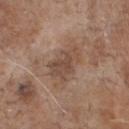Q: Was a biopsy performed?
A: catalogued during a skin exam; not biopsied
Q: What did automated image analysis measure?
A: an area of roughly 9.5 mm² and a shape-asymmetry score of about 0.35 (0 = symmetric); a mean CIELAB color near L≈47 a*≈17 b*≈26, about 8 CIELAB-L* units darker than the surrounding skin, and a normalized border contrast of about 6.5; a nevus-likeness score of about 0/100 and a lesion-detection confidence of about 100/100
Q: Lesion size?
A: about 4 mm
Q: How was the tile lit?
A: white-light
Q: What kind of image is this?
A: ~15 mm tile from a whole-body skin photo
Q: What are the patient's age and sex?
A: male, aged 68–72
Q: Where on the body is the lesion?
A: the front of the torso